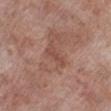Assessment: The lesion was tiled from a total-body skin photograph and was not biopsied. Image and clinical context: A 15 mm close-up tile from a total-body photography series done for melanoma screening. An algorithmic analysis of the crop reported a lesion area of about 3 mm², an outline eccentricity of about 0.95 (0 = round, 1 = elongated), and two-axis asymmetry of about 0.35. The lesion's longest dimension is about 3 mm. The tile uses white-light illumination. A male patient, in their mid- to late 70s. Located on the right lower leg.